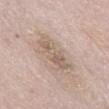| feature | finding |
|---|---|
| follow-up | catalogued during a skin exam; not biopsied |
| tile lighting | white-light illumination |
| patient | male, aged around 75 |
| size | ~5 mm (longest diameter) |
| image source | 15 mm crop, total-body photography |
| anatomic site | the abdomen |